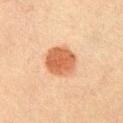Q: Was a biopsy performed?
A: catalogued during a skin exam; not biopsied
Q: What are the patient's age and sex?
A: male, roughly 60 years of age
Q: What is the anatomic site?
A: the right upper arm
Q: How large is the lesion?
A: ~4.5 mm (longest diameter)
Q: What kind of image is this?
A: ~15 mm tile from a whole-body skin photo
Q: What lighting was used for the tile?
A: cross-polarized